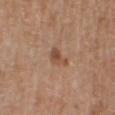Assessment: The lesion was photographed on a routine skin check and not biopsied; there is no pathology result. Acquisition and patient details: Cropped from a total-body skin-imaging series; the visible field is about 15 mm. The lesion is on the chest. This is a white-light tile. The total-body-photography lesion software estimated an area of roughly 4 mm², an outline eccentricity of about 0.8 (0 = round, 1 = elongated), and a symmetry-axis asymmetry near 0.45. The software also gave a mean CIELAB color near L≈49 a*≈21 b*≈30 and roughly 9 lightness units darker than nearby skin. Approximately 3 mm at its widest. The subject is a male approximately 70 years of age.